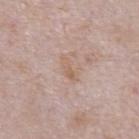Assessment:
The lesion was tiled from a total-body skin photograph and was not biopsied.
Image and clinical context:
A male patient aged approximately 60. A 15 mm crop from a total-body photograph taken for skin-cancer surveillance. Imaged with white-light lighting. The lesion's longest dimension is about 3 mm. From the abdomen.